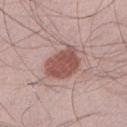No biopsy was performed on this lesion — it was imaged during a full skin examination and was not determined to be concerning. A male subject roughly 25 years of age. Longest diameter approximately 4.5 mm. Captured under white-light illumination. A 15 mm close-up extracted from a 3D total-body photography capture. The lesion is located on the leg.